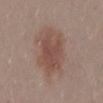The lesion was photographed on a routine skin check and not biopsied; there is no pathology result.
Approximately 7 mm at its widest.
An algorithmic analysis of the crop reported a footprint of about 22 mm², a shape eccentricity near 0.8, and two-axis asymmetry of about 0.25. The analysis additionally found border irregularity of about 3.5 on a 0–10 scale, a color-variation rating of about 4/10, and radial color variation of about 1.
The subject is a male aged approximately 30.
Located on the mid back.
A close-up tile cropped from a whole-body skin photograph, about 15 mm across.
Imaged with white-light lighting.